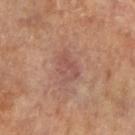{
  "biopsy_status": "not biopsied; imaged during a skin examination",
  "automated_metrics": {
    "cielab_L": 50,
    "cielab_a": 22,
    "cielab_b": 24,
    "vs_skin_darker_L": 7.0,
    "vs_skin_contrast_norm": 5.5,
    "color_variation_0_10": 3.0,
    "nevus_likeness_0_100": 0,
    "lesion_detection_confidence_0_100": 100
  },
  "image": {
    "source": "total-body photography crop",
    "field_of_view_mm": 15
  },
  "patient": {
    "sex": "female",
    "age_approx": 65
  },
  "site": "left arm",
  "lesion_size": {
    "long_diameter_mm_approx": 4.0
  }
}